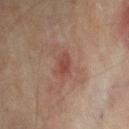Impression:
No biopsy was performed on this lesion — it was imaged during a full skin examination and was not determined to be concerning.
Acquisition and patient details:
The tile uses cross-polarized illumination. The lesion is on the right upper arm. A male patient, aged 73–77. A region of skin cropped from a whole-body photographic capture, roughly 15 mm wide. Measured at roughly 3 mm in maximum diameter.